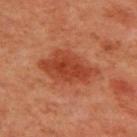Impression:
Part of a total-body skin-imaging series; this lesion was reviewed on a skin check and was not flagged for biopsy.
Image and clinical context:
A roughly 15 mm field-of-view crop from a total-body skin photograph. The patient is a male aged 58–62. Measured at roughly 7 mm in maximum diameter. From the front of the torso. The total-body-photography lesion software estimated an eccentricity of roughly 0.8. And it measured border irregularity of about 3 on a 0–10 scale and peripheral color asymmetry of about 1. The analysis additionally found a nevus-likeness score of about 75/100 and a detector confidence of about 100 out of 100 that the crop contains a lesion. The tile uses cross-polarized illumination.